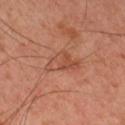– lesion size — ≈4 mm
– illumination — cross-polarized
– TBP lesion metrics — an outline eccentricity of about 0.8 (0 = round, 1 = elongated) and a symmetry-axis asymmetry near 0.45; a mean CIELAB color near L≈46 a*≈24 b*≈31, roughly 7 lightness units darker than nearby skin, and a lesion-to-skin contrast of about 5.5 (normalized; higher = more distinct); a border-irregularity rating of about 5/10 and a color-variation rating of about 3/10; an automated nevus-likeness rating near 5 out of 100 and lesion-presence confidence of about 100/100
– acquisition — 15 mm crop, total-body photography
– body site — the right upper arm
– subject — male, in their mid-40s